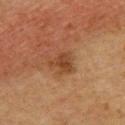Impression: Captured during whole-body skin photography for melanoma surveillance; the lesion was not biopsied. Acquisition and patient details: The tile uses cross-polarized illumination. A female patient about 60 years old. This image is a 15 mm lesion crop taken from a total-body photograph. The lesion is on the upper back. The lesion-visualizer software estimated lesion-presence confidence of about 100/100. The lesion's longest dimension is about 3 mm.